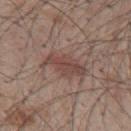Imaged during a routine full-body skin examination; the lesion was not biopsied and no histopathology is available. Imaged with white-light lighting. A 15 mm crop from a total-body photograph taken for skin-cancer surveillance. Located on the back. A male subject roughly 50 years of age.